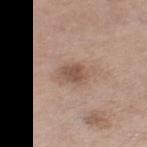Captured during whole-body skin photography for melanoma surveillance; the lesion was not biopsied. Captured under white-light illumination. Located on the leg. The recorded lesion diameter is about 3 mm. The subject is a female in their mid- to late 50s. A 15 mm close-up tile from a total-body photography series done for melanoma screening.